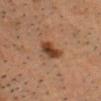The lesion was photographed on a routine skin check and not biopsied; there is no pathology result. Captured under cross-polarized illumination. A male patient, aged around 65. Cropped from a whole-body photographic skin survey; the tile spans about 15 mm. The lesion is located on the chest.